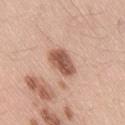biopsy status: total-body-photography surveillance lesion; no biopsy | subject: male, in their mid- to late 50s | site: the right thigh | image: 15 mm crop, total-body photography | lesion size: about 4 mm.